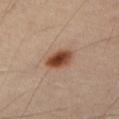notes: no biopsy performed (imaged during a skin exam)
location: the abdomen
automated lesion analysis: an area of roughly 7.5 mm², an eccentricity of roughly 0.75, and a shape-asymmetry score of about 0.15 (0 = symmetric); a within-lesion color-variation index near 6/10 and radial color variation of about 1.5; a nevus-likeness score of about 100/100 and a lesion-detection confidence of about 100/100
lesion diameter: ≈3.5 mm
imaging modality: ~15 mm tile from a whole-body skin photo
tile lighting: cross-polarized illumination
subject: male, about 65 years old